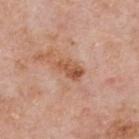biopsy status — imaged on a skin check; not biopsied | patient — male, roughly 75 years of age | automated metrics — an average lesion color of about L≈55 a*≈24 b*≈33 (CIELAB) and about 10 CIELAB-L* units darker than the surrounding skin; a border-irregularity rating of about 3.5/10 and radial color variation of about 2 | location — the upper back | acquisition — ~15 mm crop, total-body skin-cancer survey | size — ~3.5 mm (longest diameter) | tile lighting — white-light illumination.